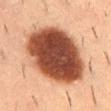Assessment: The lesion was tiled from a total-body skin photograph and was not biopsied. Acquisition and patient details: This is a cross-polarized tile. A male patient approximately 55 years of age. A 15 mm close-up tile from a total-body photography series done for melanoma screening. The recorded lesion diameter is about 9 mm. The lesion is located on the lower back.